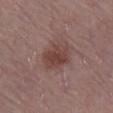Part of a total-body skin-imaging series; this lesion was reviewed on a skin check and was not flagged for biopsy. A male patient aged 73–77. Cropped from a whole-body photographic skin survey; the tile spans about 15 mm. Measured at roughly 4 mm in maximum diameter. The lesion is located on the right lower leg. The tile uses white-light illumination. An algorithmic analysis of the crop reported a within-lesion color-variation index near 3/10 and radial color variation of about 1.